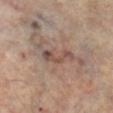notes = total-body-photography surveillance lesion; no biopsy
image source = total-body-photography crop, ~15 mm field of view
patient = female, aged around 60
site = the right lower leg